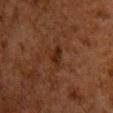| feature | finding |
|---|---|
| imaging modality | ~15 mm tile from a whole-body skin photo |
| site | the chest |
| illumination | cross-polarized illumination |
| subject | male, aged 58–62 |
| diameter | ~2.5 mm (longest diameter) |